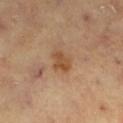Recorded during total-body skin imaging; not selected for excision or biopsy. Approximately 2.5 mm at its widest. On the left lower leg. A female subject, in their 70s. A 15 mm close-up extracted from a 3D total-body photography capture. The tile uses cross-polarized illumination.